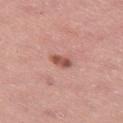workup: total-body-photography surveillance lesion; no biopsy
site: the leg
lesion size: ~2.5 mm (longest diameter)
patient: female, about 45 years old
lighting: white-light illumination
imaging modality: total-body-photography crop, ~15 mm field of view